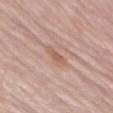<lesion>
  <biopsy_status>not biopsied; imaged during a skin examination</biopsy_status>
  <image>
    <source>total-body photography crop</source>
    <field_of_view_mm>15</field_of_view_mm>
  </image>
  <automated_metrics>
    <area_mm2_approx>2.5</area_mm2_approx>
    <eccentricity>0.9</eccentricity>
    <shape_asymmetry>0.35</shape_asymmetry>
    <vs_skin_darker_L>8.0</vs_skin_darker_L>
    <vs_skin_contrast_norm>6.5</vs_skin_contrast_norm>
    <border_irregularity_0_10>3.5</border_irregularity_0_10>
    <color_variation_0_10>0.0</color_variation_0_10>
    <nevus_likeness_0_100>0</nevus_likeness_0_100>
  </automated_metrics>
  <lighting>white-light</lighting>
  <patient>
    <sex>male</sex>
    <age_approx>85+</age_approx>
  </patient>
  <site>left thigh</site>
  <lesion_size>
    <long_diameter_mm_approx>2.5</long_diameter_mm_approx>
  </lesion_size>
</lesion>